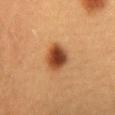biopsy status = catalogued during a skin exam; not biopsied
imaging modality = ~15 mm tile from a whole-body skin photo
illumination = cross-polarized
patient = female, aged around 30
body site = the abdomen